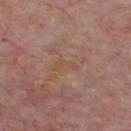biopsy_status: not biopsied; imaged during a skin examination
automated_metrics:
  eccentricity: 0.9
  shape_asymmetry: 0.45
  cielab_L: 51
  cielab_a: 18
  cielab_b: 29
  vs_skin_darker_L: 4.0
  vs_skin_contrast_norm: 4.5
  border_irregularity_0_10: 4.5
  color_variation_0_10: 0.5
  peripheral_color_asymmetry: 0.0
  lesion_detection_confidence_0_100: 90
lighting: white-light
site: chest
image:
  source: total-body photography crop
  field_of_view_mm: 15
patient:
  sex: male
  age_approx: 75